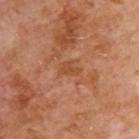Recorded during total-body skin imaging; not selected for excision or biopsy.
This image is a 15 mm lesion crop taken from a total-body photograph.
Automated image analysis of the tile measured an area of roughly 3.5 mm², an outline eccentricity of about 0.75 (0 = round, 1 = elongated), and two-axis asymmetry of about 0.45. The software also gave a border-irregularity index near 4/10 and peripheral color asymmetry of about 0.5.
About 2.5 mm across.
A male patient, aged 68 to 72.
From the upper back.
Imaged with cross-polarized lighting.